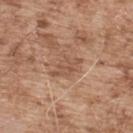- follow-up · imaged on a skin check; not biopsied
- image · ~15 mm crop, total-body skin-cancer survey
- subject · male, roughly 55 years of age
- body site · the upper back
- size · ≈3.5 mm